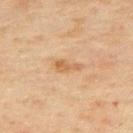{"biopsy_status": "not biopsied; imaged during a skin examination", "site": "upper back", "patient": {"sex": "male", "age_approx": 45}, "image": {"source": "total-body photography crop", "field_of_view_mm": 15}, "lesion_size": {"long_diameter_mm_approx": 3.0}}